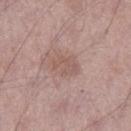The lesion was tiled from a total-body skin photograph and was not biopsied.
A close-up tile cropped from a whole-body skin photograph, about 15 mm across.
A male patient aged 48–52.
Imaged with white-light lighting.
Approximately 3 mm at its widest.
The lesion-visualizer software estimated two-axis asymmetry of about 0.25. And it measured border irregularity of about 3.5 on a 0–10 scale, a color-variation rating of about 1.5/10, and peripheral color asymmetry of about 0.5.
The lesion is on the leg.